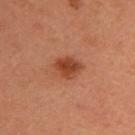Impression: The lesion was photographed on a routine skin check and not biopsied; there is no pathology result. Acquisition and patient details: The lesion is on the upper back. A close-up tile cropped from a whole-body skin photograph, about 15 mm across. The lesion's longest dimension is about 3.5 mm. A male patient roughly 35 years of age. Automated image analysis of the tile measured a footprint of about 7 mm², an outline eccentricity of about 0.65 (0 = round, 1 = elongated), and a shape-asymmetry score of about 0.15 (0 = symmetric). The software also gave about 10 CIELAB-L* units darker than the surrounding skin and a normalized lesion–skin contrast near 8. The analysis additionally found an automated nevus-likeness rating near 95 out of 100.